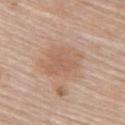Located on the upper back.
This is a white-light tile.
A female subject about 65 years old.
A 15 mm close-up extracted from a 3D total-body photography capture.
Longest diameter approximately 5 mm.